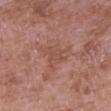- workup: catalogued during a skin exam; not biopsied
- imaging modality: ~15 mm crop, total-body skin-cancer survey
- automated metrics: an area of roughly 7.5 mm² and an eccentricity of roughly 0.55; an average lesion color of about L≈50 a*≈23 b*≈26 (CIELAB) and a lesion-to-skin contrast of about 4.5 (normalized; higher = more distinct); border irregularity of about 7 on a 0–10 scale and radial color variation of about 1; a lesion-detection confidence of about 100/100
- tile lighting: white-light
- size: about 4 mm
- patient: female, about 70 years old
- location: the right upper arm